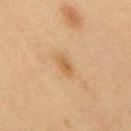Part of a total-body skin-imaging series; this lesion was reviewed on a skin check and was not flagged for biopsy. A male subject aged around 45. A lesion tile, about 15 mm wide, cut from a 3D total-body photograph. Captured under cross-polarized illumination. Measured at roughly 3 mm in maximum diameter. Located on the upper back.